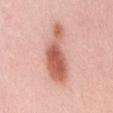Notes:
– notes — imaged on a skin check; not biopsied
– site — the mid back
– subject — female, aged around 65
– imaging modality — total-body-photography crop, ~15 mm field of view
– diameter — about 8 mm